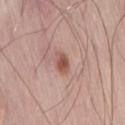A male subject, approximately 60 years of age. Cropped from a total-body skin-imaging series; the visible field is about 15 mm. Located on the lower back.